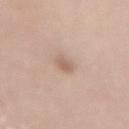<lesion>
  <biopsy_status>not biopsied; imaged during a skin examination</biopsy_status>
</lesion>